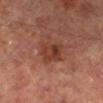Captured during whole-body skin photography for melanoma surveillance; the lesion was not biopsied. This is a cross-polarized tile. A region of skin cropped from a whole-body photographic capture, roughly 15 mm wide. The lesion-visualizer software estimated border irregularity of about 2 on a 0–10 scale, internal color variation of about 5 on a 0–10 scale, and a peripheral color-asymmetry measure near 2. A male subject, roughly 65 years of age. The lesion's longest dimension is about 4 mm. The lesion is on the right lower leg.